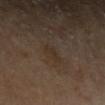follow-up — catalogued during a skin exam; not biopsied
illumination — cross-polarized illumination
size — ~2.5 mm (longest diameter)
patient — male, aged 68 to 72
anatomic site — the left upper arm
image — ~15 mm tile from a whole-body skin photo
image-analysis metrics — a nevus-likeness score of about 0/100 and a lesion-detection confidence of about 100/100